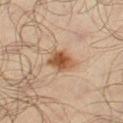The tile uses cross-polarized illumination.
A roughly 15 mm field-of-view crop from a total-body skin photograph.
From the left thigh.
About 3.5 mm across.
A male subject aged around 65.